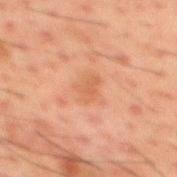* notes — catalogued during a skin exam; not biopsied
* image source — ~15 mm tile from a whole-body skin photo
* site — the back
* patient — male, aged 48 to 52
* automated metrics — a footprint of about 4 mm², an outline eccentricity of about 0.65 (0 = round, 1 = elongated), and a symmetry-axis asymmetry near 0.4; a lesion color around L≈47 a*≈21 b*≈30 in CIELAB, about 6 CIELAB-L* units darker than the surrounding skin, and a normalized lesion–skin contrast near 5; a border-irregularity rating of about 4/10, internal color variation of about 1.5 on a 0–10 scale, and a peripheral color-asymmetry measure near 0.5
* illumination — cross-polarized illumination